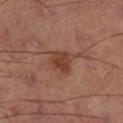No biopsy was performed on this lesion — it was imaged during a full skin examination and was not determined to be concerning. On the right thigh. Imaged with cross-polarized lighting. A region of skin cropped from a whole-body photographic capture, roughly 15 mm wide.